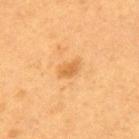follow-up=no biopsy performed (imaged during a skin exam)
size=about 2.5 mm
imaging modality=total-body-photography crop, ~15 mm field of view
site=the upper back
tile lighting=cross-polarized illumination
subject=female, aged 38 to 42